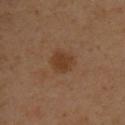<lesion>
<lighting>cross-polarized</lighting>
<patient>
  <sex>male</sex>
  <age_approx>40</age_approx>
</patient>
<site>upper back</site>
<image>
  <source>total-body photography crop</source>
  <field_of_view_mm>15</field_of_view_mm>
</image>
</lesion>